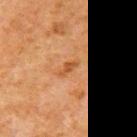Findings:
• subject · male, about 80 years old
• body site · the right upper arm
• image source · ~15 mm tile from a whole-body skin photo